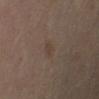Recorded during total-body skin imaging; not selected for excision or biopsy.
A female patient, aged approximately 50.
Cropped from a total-body skin-imaging series; the visible field is about 15 mm.
The tile uses cross-polarized illumination.
The lesion is located on the arm.
Automated tile analysis of the lesion measured a footprint of about 3 mm², an eccentricity of roughly 0.9, and a symmetry-axis asymmetry near 0.3. The software also gave a lesion color around L≈37 a*≈12 b*≈22 in CIELAB. The analysis additionally found a within-lesion color-variation index near 1/10 and radial color variation of about 0.5. It also reported a classifier nevus-likeness of about 0/100 and lesion-presence confidence of about 100/100.
Approximately 3 mm at its widest.